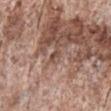Q: Is there a histopathology result?
A: imaged on a skin check; not biopsied
Q: Patient demographics?
A: female, aged 43–47
Q: How large is the lesion?
A: about 2 mm
Q: What is the imaging modality?
A: ~15 mm tile from a whole-body skin photo
Q: Where on the body is the lesion?
A: the back
Q: How was the tile lit?
A: white-light